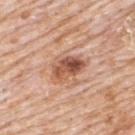Background:
From the upper back. The tile uses white-light illumination. A lesion tile, about 15 mm wide, cut from a 3D total-body photograph. Measured at roughly 4.5 mm in maximum diameter. The subject is a male roughly 80 years of age.